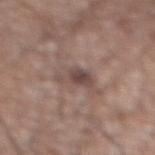workup: total-body-photography surveillance lesion; no biopsy
anatomic site: the abdomen
subject: male, in their mid- to late 70s
image-analysis metrics: an area of roughly 5.5 mm², an eccentricity of roughly 0.85, and two-axis asymmetry of about 0.35
image source: ~15 mm tile from a whole-body skin photo